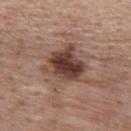Impression: The lesion was photographed on a routine skin check and not biopsied; there is no pathology result.